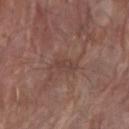* workup: catalogued during a skin exam; not biopsied
* illumination: white-light
* image: ~15 mm tile from a whole-body skin photo
* patient: male, aged 68–72
* diameter: ~2.5 mm (longest diameter)
* body site: the right forearm
* TBP lesion metrics: border irregularity of about 4.5 on a 0–10 scale, a color-variation rating of about 0/10, and a peripheral color-asymmetry measure near 0